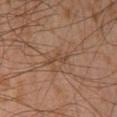Q: Was this lesion biopsied?
A: total-body-photography surveillance lesion; no biopsy
Q: How large is the lesion?
A: about 3 mm
Q: Illumination type?
A: cross-polarized
Q: What is the anatomic site?
A: the right lower leg
Q: How was this image acquired?
A: total-body-photography crop, ~15 mm field of view
Q: Who is the patient?
A: male, aged 43 to 47